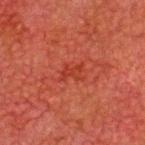No biopsy was performed on this lesion — it was imaged during a full skin examination and was not determined to be concerning. The lesion's longest dimension is about 2.5 mm. A region of skin cropped from a whole-body photographic capture, roughly 15 mm wide. Automated image analysis of the tile measured a lesion color around L≈31 a*≈31 b*≈30 in CIELAB, roughly 5 lightness units darker than nearby skin, and a normalized border contrast of about 5.5. From the head or neck. The subject is a male approximately 60 years of age. Imaged with cross-polarized lighting.